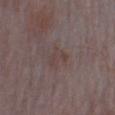No biopsy was performed on this lesion — it was imaged during a full skin examination and was not determined to be concerning.
A 15 mm crop from a total-body photograph taken for skin-cancer surveillance.
The lesion-visualizer software estimated a lesion area of about 4 mm² and a symmetry-axis asymmetry near 0.45. It also reported internal color variation of about 0.5 on a 0–10 scale and a peripheral color-asymmetry measure near 0.
The subject is a male about 65 years old.
From the left upper arm.
This is a white-light tile.